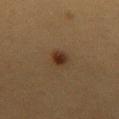| field | value |
|---|---|
| biopsy status | total-body-photography surveillance lesion; no biopsy |
| site | the chest |
| lighting | cross-polarized illumination |
| diameter | ≈2.5 mm |
| patient | female, approximately 20 years of age |
| acquisition | total-body-photography crop, ~15 mm field of view |